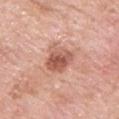The lesion was tiled from a total-body skin photograph and was not biopsied. The recorded lesion diameter is about 3.5 mm. Cropped from a whole-body photographic skin survey; the tile spans about 15 mm. The tile uses white-light illumination. The lesion-visualizer software estimated a lesion color around L≈57 a*≈26 b*≈29 in CIELAB. It also reported an automated nevus-likeness rating near 50 out of 100 and a lesion-detection confidence of about 100/100. A male patient aged 53 to 57. The lesion is on the chest.